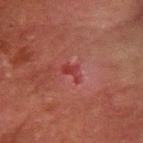<lesion>
  <biopsy_status>not biopsied; imaged during a skin examination</biopsy_status>
  <lighting>cross-polarized</lighting>
  <image>
    <source>total-body photography crop</source>
    <field_of_view_mm>15</field_of_view_mm>
  </image>
  <patient>
    <sex>male</sex>
    <age_approx>80</age_approx>
  </patient>
  <site>left forearm</site>
  <lesion_size>
    <long_diameter_mm_approx>2.5</long_diameter_mm_approx>
  </lesion_size>
  <automated_metrics>
    <vs_skin_darker_L>6.0</vs_skin_darker_L>
    <vs_skin_contrast_norm>5.5</vs_skin_contrast_norm>
    <nevus_likeness_0_100>0</nevus_likeness_0_100>
  </automated_metrics>
</lesion>